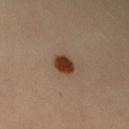Clinical impression: Recorded during total-body skin imaging; not selected for excision or biopsy. Acquisition and patient details: The subject is a female aged 33 to 37. The lesion-visualizer software estimated a lesion area of about 5.5 mm², an eccentricity of roughly 0.6, and a shape-asymmetry score of about 0.15 (0 = symmetric). And it measured a lesion color around L≈37 a*≈19 b*≈28 in CIELAB and about 15 CIELAB-L* units darker than the surrounding skin. It also reported a border-irregularity rating of about 1/10, internal color variation of about 3 on a 0–10 scale, and peripheral color asymmetry of about 1. A region of skin cropped from a whole-body photographic capture, roughly 15 mm wide. The lesion is on the arm. About 3 mm across. This is a cross-polarized tile.